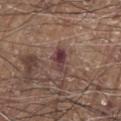follow-up=imaged on a skin check; not biopsied
patient=male, roughly 80 years of age
site=the chest
acquisition=~15 mm crop, total-body skin-cancer survey
lighting=white-light
diameter=~3.5 mm (longest diameter)